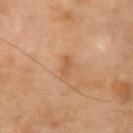This lesion was catalogued during total-body skin photography and was not selected for biopsy.
A lesion tile, about 15 mm wide, cut from a 3D total-body photograph.
Automated image analysis of the tile measured a footprint of about 3 mm², an outline eccentricity of about 0.9 (0 = round, 1 = elongated), and two-axis asymmetry of about 0.3. And it measured an average lesion color of about L≈55 a*≈22 b*≈37 (CIELAB), a lesion–skin lightness drop of about 7, and a normalized lesion–skin contrast near 6. The analysis additionally found a border-irregularity index near 3/10, a color-variation rating of about 0/10, and radial color variation of about 0. It also reported a nevus-likeness score of about 0/100 and lesion-presence confidence of about 100/100.
From the left upper arm.
The subject is a male aged approximately 70.
Longest diameter approximately 3 mm.